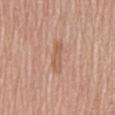notes = total-body-photography surveillance lesion; no biopsy
image = 15 mm crop, total-body photography
subject = male, aged 68 to 72
site = the mid back